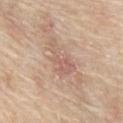Case summary:
– workup — no biopsy performed (imaged during a skin exam)
– subject — male, in their mid- to late 60s
– imaging modality — total-body-photography crop, ~15 mm field of view
– anatomic site — the chest